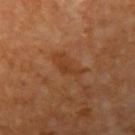Q: Was a biopsy performed?
A: imaged on a skin check; not biopsied
Q: How was the tile lit?
A: cross-polarized
Q: What is the imaging modality?
A: ~15 mm crop, total-body skin-cancer survey
Q: Patient demographics?
A: in their mid-60s
Q: Automated lesion metrics?
A: an average lesion color of about L≈38 a*≈24 b*≈35 (CIELAB), about 7 CIELAB-L* units darker than the surrounding skin, and a normalized border contrast of about 6
Q: Lesion location?
A: the left upper arm